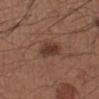| feature | finding |
|---|---|
| follow-up | catalogued during a skin exam; not biopsied |
| TBP lesion metrics | a footprint of about 6 mm² and an outline eccentricity of about 0.65 (0 = round, 1 = elongated); a mean CIELAB color near L≈36 a*≈20 b*≈24, about 10 CIELAB-L* units darker than the surrounding skin, and a lesion-to-skin contrast of about 8.5 (normalized; higher = more distinct) |
| lesion size | ~3 mm (longest diameter) |
| imaging modality | ~15 mm tile from a whole-body skin photo |
| tile lighting | white-light |
| subject | male, aged 53–57 |
| body site | the arm |